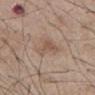Impression: The lesion was tiled from a total-body skin photograph and was not biopsied. Clinical summary: A region of skin cropped from a whole-body photographic capture, roughly 15 mm wide. A male subject in their mid- to late 50s. Automated tile analysis of the lesion measured a lesion color around L≈53 a*≈17 b*≈27 in CIELAB, about 7 CIELAB-L* units darker than the surrounding skin, and a normalized lesion–skin contrast near 5.5. Located on the chest. Approximately 3.5 mm at its widest.